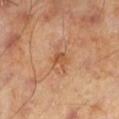– biopsy status · imaged on a skin check; not biopsied
– subject · male, in their mid-40s
– diameter · about 3.5 mm
– imaging modality · ~15 mm tile from a whole-body skin photo
– location · the left lower leg
– tile lighting · cross-polarized illumination